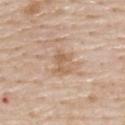Q: Is there a histopathology result?
A: no biopsy performed (imaged during a skin exam)
Q: How was this image acquired?
A: 15 mm crop, total-body photography
Q: Lesion location?
A: the upper back
Q: Lesion size?
A: about 3 mm
Q: How was the tile lit?
A: white-light illumination
Q: Patient demographics?
A: male, aged 78–82
Q: What did automated image analysis measure?
A: an outline eccentricity of about 0.5 (0 = round, 1 = elongated) and a symmetry-axis asymmetry near 0.35; a classifier nevus-likeness of about 0/100 and a lesion-detection confidence of about 100/100